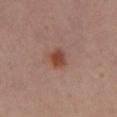Q: Is there a histopathology result?
A: imaged on a skin check; not biopsied
Q: Patient demographics?
A: female, aged 38–42
Q: Where on the body is the lesion?
A: the left lower leg
Q: What is the imaging modality?
A: total-body-photography crop, ~15 mm field of view
Q: Lesion size?
A: about 2.5 mm